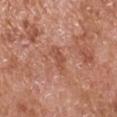notes: total-body-photography surveillance lesion; no biopsy
patient: male, approximately 65 years of age
anatomic site: the left upper arm
lighting: white-light illumination
imaging modality: ~15 mm crop, total-body skin-cancer survey
diameter: ~3.5 mm (longest diameter)